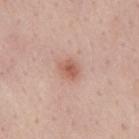| field | value |
|---|---|
| notes | catalogued during a skin exam; not biopsied |
| automated lesion analysis | a lesion area of about 4 mm², an outline eccentricity of about 0.6 (0 = round, 1 = elongated), and two-axis asymmetry of about 0.25; an average lesion color of about L≈58 a*≈23 b*≈29 (CIELAB), roughly 10 lightness units darker than nearby skin, and a lesion-to-skin contrast of about 7 (normalized; higher = more distinct) |
| body site | the mid back |
| subject | male, aged around 55 |
| lesion size | ~2.5 mm (longest diameter) |
| lighting | white-light |
| imaging modality | 15 mm crop, total-body photography |